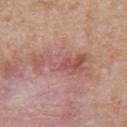Impression:
Imaged during a routine full-body skin examination; the lesion was not biopsied and no histopathology is available.
Acquisition and patient details:
Located on the front of the torso. The subject is a male roughly 50 years of age. A lesion tile, about 15 mm wide, cut from a 3D total-body photograph.